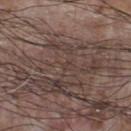No biopsy was performed on this lesion — it was imaged during a full skin examination and was not determined to be concerning.
Automated image analysis of the tile measured an area of roughly 0.5 mm², an outline eccentricity of about 0.8 (0 = round, 1 = elongated), and two-axis asymmetry of about 0.6. It also reported an automated nevus-likeness rating near 0 out of 100 and lesion-presence confidence of about 5/100.
Cropped from a total-body skin-imaging series; the visible field is about 15 mm.
Approximately 1 mm at its widest.
The lesion is located on the chest.
The patient is a male aged approximately 75.
Captured under white-light illumination.